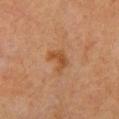Clinical impression: Recorded during total-body skin imaging; not selected for excision or biopsy. Background: A female subject, aged 38–42. Longest diameter approximately 3 mm. Captured under cross-polarized illumination. The lesion is located on the chest. A roughly 15 mm field-of-view crop from a total-body skin photograph. Automated tile analysis of the lesion measured a lesion area of about 4.5 mm², an outline eccentricity of about 0.75 (0 = round, 1 = elongated), and a symmetry-axis asymmetry near 0.45. And it measured roughly 7 lightness units darker than nearby skin and a lesion-to-skin contrast of about 7 (normalized; higher = more distinct). It also reported border irregularity of about 4.5 on a 0–10 scale, a color-variation rating of about 1.5/10, and radial color variation of about 0.5. The analysis additionally found a classifier nevus-likeness of about 5/100.